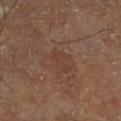Clinical impression:
The lesion was tiled from a total-body skin photograph and was not biopsied.
Acquisition and patient details:
From the right lower leg. Measured at roughly 3 mm in maximum diameter. The tile uses cross-polarized illumination. The lesion-visualizer software estimated an area of roughly 3.5 mm², an outline eccentricity of about 0.85 (0 = round, 1 = elongated), and a shape-asymmetry score of about 0.5 (0 = symmetric). It also reported border irregularity of about 5.5 on a 0–10 scale and peripheral color asymmetry of about 0. The analysis additionally found an automated nevus-likeness rating near 0 out of 100 and lesion-presence confidence of about 100/100. The patient is a male aged approximately 70. A region of skin cropped from a whole-body photographic capture, roughly 15 mm wide.